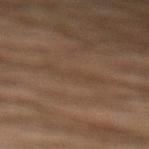The lesion was photographed on a routine skin check and not biopsied; there is no pathology result. The subject is a male approximately 45 years of age. Cropped from a whole-body photographic skin survey; the tile spans about 15 mm. Captured under cross-polarized illumination. The lesion is located on the left lower leg.